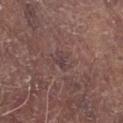No biopsy was performed on this lesion — it was imaged during a full skin examination and was not determined to be concerning. A male patient aged approximately 80. Captured under white-light illumination. A 15 mm close-up extracted from a 3D total-body photography capture. Automated image analysis of the tile measured a lesion area of about 3 mm², an outline eccentricity of about 0.8 (0 = round, 1 = elongated), and two-axis asymmetry of about 0.45. And it measured an automated nevus-likeness rating near 0 out of 100. Located on the chest.